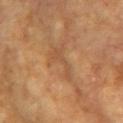Case summary:
• follow-up — catalogued during a skin exam; not biopsied
• subject — female, aged 73–77
• site — the left upper arm
• imaging modality — ~15 mm tile from a whole-body skin photo